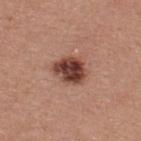Q: Was this lesion biopsied?
A: catalogued during a skin exam; not biopsied
Q: What is the imaging modality?
A: total-body-photography crop, ~15 mm field of view
Q: Where on the body is the lesion?
A: the upper back
Q: Automated lesion metrics?
A: internal color variation of about 6 on a 0–10 scale and peripheral color asymmetry of about 2; an automated nevus-likeness rating near 85 out of 100 and a lesion-detection confidence of about 100/100
Q: Who is the patient?
A: male, aged around 40
Q: Illumination type?
A: white-light illumination